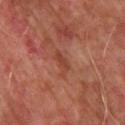Clinical impression: The lesion was tiled from a total-body skin photograph and was not biopsied. Background: On the upper back. A 15 mm crop from a total-body photograph taken for skin-cancer surveillance. A male patient, aged 63–67. About 3 mm across. The total-body-photography lesion software estimated an average lesion color of about L≈41 a*≈26 b*≈30 (CIELAB), a lesion–skin lightness drop of about 7, and a normalized border contrast of about 6. And it measured a border-irregularity index near 6/10, a color-variation rating of about 1/10, and a peripheral color-asymmetry measure near 0.5. The software also gave a nevus-likeness score of about 0/100.